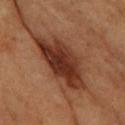| field | value |
|---|---|
| biopsy status | no biopsy performed (imaged during a skin exam) |
| automated lesion analysis | an average lesion color of about L≈32 a*≈24 b*≈29 (CIELAB), about 14 CIELAB-L* units darker than the surrounding skin, and a normalized lesion–skin contrast near 12; an automated nevus-likeness rating near 80 out of 100 and a lesion-detection confidence of about 100/100 |
| body site | the arm |
| image | ~15 mm crop, total-body skin-cancer survey |
| subject | female, in their mid-60s |
| illumination | cross-polarized |